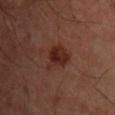{
  "biopsy_status": "not biopsied; imaged during a skin examination",
  "lighting": "cross-polarized",
  "site": "front of the torso",
  "patient": {
    "sex": "male",
    "age_approx": 50
  },
  "image": {
    "source": "total-body photography crop",
    "field_of_view_mm": 15
  }
}